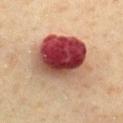Captured during whole-body skin photography for melanoma surveillance; the lesion was not biopsied.
A lesion tile, about 15 mm wide, cut from a 3D total-body photograph.
Captured under cross-polarized illumination.
The lesion is on the chest.
A male subject, in their 60s.